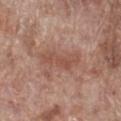The lesion was tiled from a total-body skin photograph and was not biopsied.
Longest diameter approximately 5.5 mm.
From the right lower leg.
Cropped from a whole-body photographic skin survey; the tile spans about 15 mm.
Captured under white-light illumination.
A male subject about 70 years old.